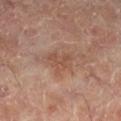follow-up = imaged on a skin check; not biopsied
imaging modality = ~15 mm tile from a whole-body skin photo
subject = male, aged 63 to 67
location = the right lower leg
TBP lesion metrics = a border-irregularity rating of about 4.5/10, a color-variation rating of about 1.5/10, and a peripheral color-asymmetry measure near 0.5; a classifier nevus-likeness of about 0/100
size = about 3 mm
tile lighting = cross-polarized